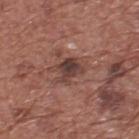| feature | finding |
|---|---|
| follow-up | imaged on a skin check; not biopsied |
| illumination | white-light |
| imaging modality | 15 mm crop, total-body photography |
| site | the upper back |
| lesion diameter | about 3 mm |
| subject | male, aged 73 to 77 |